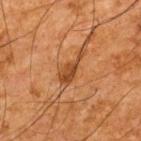Q: Was a biopsy performed?
A: no biopsy performed (imaged during a skin exam)
Q: What are the patient's age and sex?
A: male, aged approximately 65
Q: Lesion size?
A: ~4.5 mm (longest diameter)
Q: How was the tile lit?
A: cross-polarized
Q: Lesion location?
A: the upper back
Q: How was this image acquired?
A: ~15 mm crop, total-body skin-cancer survey
Q: Automated lesion metrics?
A: a border-irregularity rating of about 6.5/10 and a color-variation rating of about 2.5/10